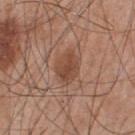The lesion was tiled from a total-body skin photograph and was not biopsied. A roughly 15 mm field-of-view crop from a total-body skin photograph. This is a white-light tile. The subject is a male in their mid- to late 50s. Located on the front of the torso.